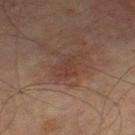workup: no biopsy performed (imaged during a skin exam) | size: about 2.5 mm | subject: male, roughly 75 years of age | acquisition: total-body-photography crop, ~15 mm field of view | anatomic site: the left thigh.